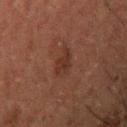Q: Is there a histopathology result?
A: total-body-photography surveillance lesion; no biopsy
Q: How large is the lesion?
A: ≈3 mm
Q: Where on the body is the lesion?
A: the arm
Q: Illumination type?
A: cross-polarized illumination
Q: What did automated image analysis measure?
A: a lesion area of about 4.5 mm², a shape eccentricity near 0.7, and a symmetry-axis asymmetry near 0.2; a peripheral color-asymmetry measure near 0.5; a nevus-likeness score of about 15/100 and a detector confidence of about 100 out of 100 that the crop contains a lesion
Q: Patient demographics?
A: male, roughly 50 years of age
Q: How was this image acquired?
A: 15 mm crop, total-body photography